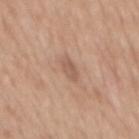The lesion was tiled from a total-body skin photograph and was not biopsied.
This image is a 15 mm lesion crop taken from a total-body photograph.
On the back.
The subject is a female in their 40s.